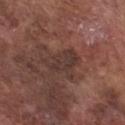Q: Was this lesion biopsied?
A: imaged on a skin check; not biopsied
Q: What is the lesion's diameter?
A: about 4.5 mm
Q: Who is the patient?
A: male, aged 73–77
Q: What is the anatomic site?
A: the chest
Q: What is the imaging modality?
A: 15 mm crop, total-body photography
Q: Automated lesion metrics?
A: a border-irregularity index near 4.5/10 and internal color variation of about 3 on a 0–10 scale; a classifier nevus-likeness of about 0/100 and a detector confidence of about 95 out of 100 that the crop contains a lesion